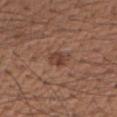Assessment:
Part of a total-body skin-imaging series; this lesion was reviewed on a skin check and was not flagged for biopsy.
Acquisition and patient details:
An algorithmic analysis of the crop reported a nevus-likeness score of about 80/100 and lesion-presence confidence of about 100/100. About 2.5 mm across. From the right upper arm. A male patient aged 53 to 57. Imaged with white-light lighting. Cropped from a total-body skin-imaging series; the visible field is about 15 mm.